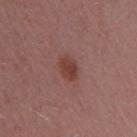<record>
  <site>right upper arm</site>
  <lesion_size>
    <long_diameter_mm_approx>3.0</long_diameter_mm_approx>
  </lesion_size>
  <image>
    <source>total-body photography crop</source>
    <field_of_view_mm>15</field_of_view_mm>
  </image>
  <lighting>white-light</lighting>
  <automated_metrics>
    <eccentricity>0.7</eccentricity>
    <shape_asymmetry>0.15</shape_asymmetry>
    <vs_skin_contrast_norm>7.5</vs_skin_contrast_norm>
  </automated_metrics>
  <patient>
    <sex>female</sex>
    <age_approx>45</age_approx>
  </patient>
</record>